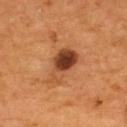Findings:
– notes · catalogued during a skin exam; not biopsied
– lesion diameter · ~4.5 mm (longest diameter)
– image · total-body-photography crop, ~15 mm field of view
– patient · male, approximately 55 years of age
– location · the upper back
– tile lighting · cross-polarized illumination